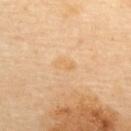The lesion was photographed on a routine skin check and not biopsied; there is no pathology result. An algorithmic analysis of the crop reported a lesion area of about 3 mm², a shape eccentricity near 0.85, and a symmetry-axis asymmetry near 0.25. It also reported a border-irregularity index near 2.5/10 and internal color variation of about 2 on a 0–10 scale. Approximately 2.5 mm at its widest. A female subject approximately 60 years of age. On the upper back. Imaged with cross-polarized lighting. A lesion tile, about 15 mm wide, cut from a 3D total-body photograph.